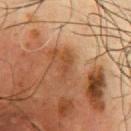Q: Was a biopsy performed?
A: imaged on a skin check; not biopsied
Q: Patient demographics?
A: male, about 60 years old
Q: What kind of image is this?
A: ~15 mm tile from a whole-body skin photo
Q: Where on the body is the lesion?
A: the chest